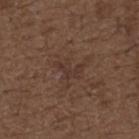Clinical summary: A male subject aged approximately 50. The tile uses white-light illumination. The recorded lesion diameter is about 3.5 mm. Automated image analysis of the tile measured a footprint of about 4.5 mm², an eccentricity of roughly 0.9, and a symmetry-axis asymmetry near 0.5. It also reported a nevus-likeness score of about 0/100 and a detector confidence of about 90 out of 100 that the crop contains a lesion. Cropped from a total-body skin-imaging series; the visible field is about 15 mm. From the upper back.